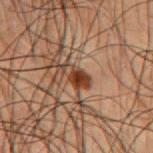biopsy status — imaged on a skin check; not biopsied | tile lighting — cross-polarized | diameter — ≈3.5 mm | patient — male, about 50 years old | site — the back | imaging modality — total-body-photography crop, ~15 mm field of view.